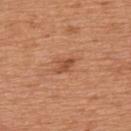notes=catalogued during a skin exam; not biopsied.